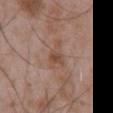Findings:
– workup · catalogued during a skin exam; not biopsied
– subject · male, in their 50s
– image-analysis metrics · a border-irregularity index near 4.5/10 and a peripheral color-asymmetry measure near 1
– lighting · white-light
– site · the mid back
– size · ≈4.5 mm
– acquisition · ~15 mm tile from a whole-body skin photo